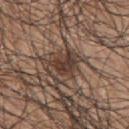Q: Is there a histopathology result?
A: catalogued during a skin exam; not biopsied
Q: What is the imaging modality?
A: 15 mm crop, total-body photography
Q: Patient demographics?
A: male, approximately 70 years of age
Q: Lesion location?
A: the arm
Q: What is the lesion's diameter?
A: ~3 mm (longest diameter)
Q: Automated lesion metrics?
A: a lesion color around L≈36 a*≈17 b*≈23 in CIELAB and about 11 CIELAB-L* units darker than the surrounding skin